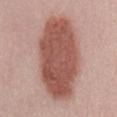<case>
<biopsy_status>not biopsied; imaged during a skin examination</biopsy_status>
<lesion_size>
  <long_diameter_mm_approx>11.0</long_diameter_mm_approx>
</lesion_size>
<lighting>white-light</lighting>
<site>mid back</site>
<patient>
  <sex>female</sex>
  <age_approx>50</age_approx>
</patient>
<image>
  <source>total-body photography crop</source>
  <field_of_view_mm>15</field_of_view_mm>
</image>
<automated_metrics>
  <area_mm2_approx>47.0</area_mm2_approx>
  <eccentricity>0.85</eccentricity>
  <shape_asymmetry>0.1</shape_asymmetry>
  <cielab_L>53</cielab_L>
  <cielab_a>24</cielab_a>
  <cielab_b>26</cielab_b>
  <nevus_likeness_0_100>100</nevus_likeness_0_100>
  <lesion_detection_confidence_0_100>100</lesion_detection_confidence_0_100>
</automated_metrics>
</case>